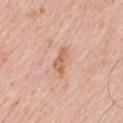Assessment:
This lesion was catalogued during total-body skin photography and was not selected for biopsy.
Context:
A male subject roughly 80 years of age. Longest diameter approximately 3.5 mm. From the chest. The tile uses white-light illumination. This image is a 15 mm lesion crop taken from a total-body photograph.